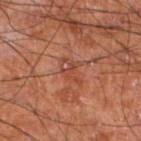The lesion was photographed on a routine skin check and not biopsied; there is no pathology result. On the leg. Imaged with cross-polarized lighting. A 15 mm crop from a total-body photograph taken for skin-cancer surveillance. A male subject aged 58 to 62. About 3 mm across.